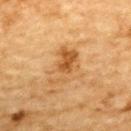This lesion was catalogued during total-body skin photography and was not selected for biopsy.
A region of skin cropped from a whole-body photographic capture, roughly 15 mm wide.
On the upper back.
A male patient, in their mid-80s or older.
This is a cross-polarized tile.
Approximately 5 mm at its widest.